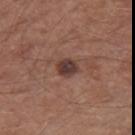| key | value |
|---|---|
| notes | imaged on a skin check; not biopsied |
| size | ≈2.5 mm |
| body site | the right thigh |
| patient | male, aged around 65 |
| automated metrics | a border-irregularity rating of about 1.5/10, internal color variation of about 3.5 on a 0–10 scale, and radial color variation of about 1; a classifier nevus-likeness of about 45/100 and a detector confidence of about 100 out of 100 that the crop contains a lesion |
| lighting | white-light illumination |
| acquisition | 15 mm crop, total-body photography |